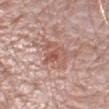Assessment:
No biopsy was performed on this lesion — it was imaged during a full skin examination and was not determined to be concerning.
Clinical summary:
The recorded lesion diameter is about 4 mm. On the left upper arm. This is a white-light tile. A male patient, aged around 65. Automated tile analysis of the lesion measured an area of roughly 8.5 mm². The analysis additionally found a mean CIELAB color near L≈56 a*≈22 b*≈25, roughly 9 lightness units darker than nearby skin, and a normalized lesion–skin contrast near 6. The software also gave a border-irregularity rating of about 4.5/10 and internal color variation of about 5.5 on a 0–10 scale. A lesion tile, about 15 mm wide, cut from a 3D total-body photograph.